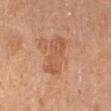<lesion>
<biopsy_status>not biopsied; imaged during a skin examination</biopsy_status>
<site>right lower leg</site>
<patient>
  <sex>female</sex>
  <age_approx>55</age_approx>
</patient>
<image>
  <source>total-body photography crop</source>
  <field_of_view_mm>15</field_of_view_mm>
</image>
</lesion>